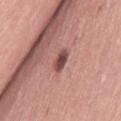notes = imaged on a skin check; not biopsied
imaging modality = ~15 mm crop, total-body skin-cancer survey
image-analysis metrics = a mean CIELAB color near L≈47 a*≈23 b*≈22, about 15 CIELAB-L* units darker than the surrounding skin, and a normalized border contrast of about 10; a nevus-likeness score of about 95/100 and a lesion-detection confidence of about 100/100
size = about 3 mm
site = the left thigh
tile lighting = white-light illumination
patient = female, in their mid- to late 60s